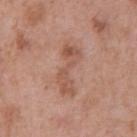Background: Cropped from a whole-body photographic skin survey; the tile spans about 15 mm. A male patient, about 70 years old. From the front of the torso.